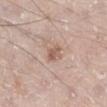{"biopsy_status": "not biopsied; imaged during a skin examination", "site": "leg", "lesion_size": {"long_diameter_mm_approx": 2.5}, "image": {"source": "total-body photography crop", "field_of_view_mm": 15}, "patient": {"sex": "male", "age_approx": 60}, "lighting": "white-light"}